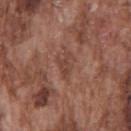| key | value |
|---|---|
| workup | no biopsy performed (imaged during a skin exam) |
| body site | the chest |
| illumination | white-light |
| imaging modality | total-body-photography crop, ~15 mm field of view |
| subject | male, in their mid- to late 70s |